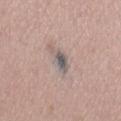Captured during whole-body skin photography for melanoma surveillance; the lesion was not biopsied. Captured under white-light illumination. Automated image analysis of the tile measured a nevus-likeness score of about 80/100 and a detector confidence of about 95 out of 100 that the crop contains a lesion. A male subject, aged 68 to 72. The lesion's longest dimension is about 3 mm. On the back. A 15 mm crop from a total-body photograph taken for skin-cancer surveillance.